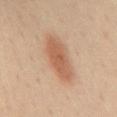Imaged during a routine full-body skin examination; the lesion was not biopsied and no histopathology is available. On the mid back. The recorded lesion diameter is about 6.5 mm. An algorithmic analysis of the crop reported a footprint of about 13 mm², an outline eccentricity of about 0.9 (0 = round, 1 = elongated), and a shape-asymmetry score of about 0.15 (0 = symmetric). The analysis additionally found a lesion color around L≈60 a*≈22 b*≈34 in CIELAB, a lesion–skin lightness drop of about 11, and a lesion-to-skin contrast of about 7.5 (normalized; higher = more distinct). It also reported an automated nevus-likeness rating near 100 out of 100. A 15 mm crop from a total-body photograph taken for skin-cancer surveillance. The patient is a female aged 43 to 47.